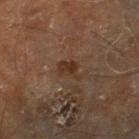workup = catalogued during a skin exam; not biopsied | illumination = cross-polarized | anatomic site = the right lower leg | imaging modality = 15 mm crop, total-body photography | subject = male, aged approximately 70 | lesion diameter = ≈3 mm | automated lesion analysis = a lesion color around L≈32 a*≈17 b*≈26 in CIELAB and a lesion–skin lightness drop of about 7; internal color variation of about 2 on a 0–10 scale and a peripheral color-asymmetry measure near 1.